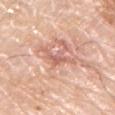Recorded during total-body skin imaging; not selected for excision or biopsy.
This image is a 15 mm lesion crop taken from a total-body photograph.
From the left arm.
A male subject aged approximately 80.
The recorded lesion diameter is about 5.5 mm.
An algorithmic analysis of the crop reported a shape eccentricity near 0.95 and a symmetry-axis asymmetry near 0.6. The analysis additionally found internal color variation of about 2 on a 0–10 scale and radial color variation of about 0.5.
The tile uses white-light illumination.